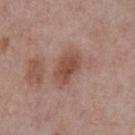Notes:
- workup · catalogued during a skin exam; not biopsied
- image · ~15 mm tile from a whole-body skin photo
- anatomic site · the chest
- patient · male, aged 73–77